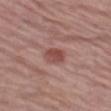{
  "biopsy_status": "not biopsied; imaged during a skin examination",
  "lighting": "white-light",
  "site": "left thigh",
  "lesion_size": {
    "long_diameter_mm_approx": 2.5
  },
  "patient": {
    "sex": "female",
    "age_approx": "85+"
  },
  "image": {
    "source": "total-body photography crop",
    "field_of_view_mm": 15
  }
}